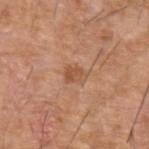Clinical impression: Part of a total-body skin-imaging series; this lesion was reviewed on a skin check and was not flagged for biopsy. Acquisition and patient details: From the left forearm. The recorded lesion diameter is about 2.5 mm. A male patient, aged approximately 75. Cropped from a whole-body photographic skin survey; the tile spans about 15 mm. This is a white-light tile.